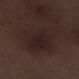workup: total-body-photography surveillance lesion; no biopsy
image: 15 mm crop, total-body photography
anatomic site: the right lower leg
lesion size: ~7 mm (longest diameter)
patient: male, aged approximately 70
tile lighting: white-light